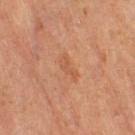biopsy status = imaged on a skin check; not biopsied
tile lighting = cross-polarized illumination
patient = female, in their 70s
lesion diameter = ~3 mm (longest diameter)
image source = ~15 mm tile from a whole-body skin photo
site = the right thigh